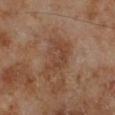The lesion was photographed on a routine skin check and not biopsied; there is no pathology result. The lesion is located on the left lower leg. Imaged with cross-polarized lighting. A male patient, aged approximately 70. Automated image analysis of the tile measured an area of roughly 11 mm², an eccentricity of roughly 0.85, and a symmetry-axis asymmetry near 0.45. The analysis additionally found a color-variation rating of about 3.5/10 and peripheral color asymmetry of about 1. A lesion tile, about 15 mm wide, cut from a 3D total-body photograph.